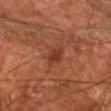biopsy status: no biopsy performed (imaged during a skin exam) | automated metrics: a lesion color around L≈37 a*≈26 b*≈32 in CIELAB, about 8 CIELAB-L* units darker than the surrounding skin, and a lesion-to-skin contrast of about 7 (normalized; higher = more distinct); a color-variation rating of about 1.5/10 and radial color variation of about 0.5; a classifier nevus-likeness of about 55/100 and a detector confidence of about 100 out of 100 that the crop contains a lesion | illumination: cross-polarized illumination | subject: male, aged around 70 | image source: ~15 mm tile from a whole-body skin photo | location: the left forearm | size: about 3.5 mm.